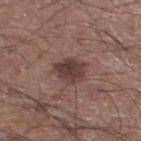Captured during whole-body skin photography for melanoma surveillance; the lesion was not biopsied.
Cropped from a total-body skin-imaging series; the visible field is about 15 mm.
A male patient aged 58–62.
On the left lower leg.
Captured under white-light illumination.